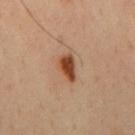automated lesion analysis: about 15 CIELAB-L* units darker than the surrounding skin and a lesion-to-skin contrast of about 11.5 (normalized; higher = more distinct); a within-lesion color-variation index near 3/10 and radial color variation of about 1; a classifier nevus-likeness of about 100/100 and lesion-presence confidence of about 100/100
subject: male, about 45 years old
acquisition: 15 mm crop, total-body photography
location: the mid back